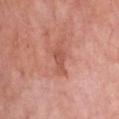Part of a total-body skin-imaging series; this lesion was reviewed on a skin check and was not flagged for biopsy.
On the front of the torso.
The patient is a male roughly 80 years of age.
Cropped from a total-body skin-imaging series; the visible field is about 15 mm.